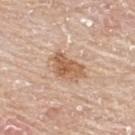Impression:
Imaged during a routine full-body skin examination; the lesion was not biopsied and no histopathology is available.
Context:
Cropped from a total-body skin-imaging series; the visible field is about 15 mm. The tile uses white-light illumination. A male subject in their mid-60s. Longest diameter approximately 4.5 mm. On the upper back.